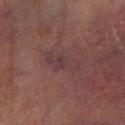Q: Is there a histopathology result?
A: no biopsy performed (imaged during a skin exam)
Q: Where on the body is the lesion?
A: the right lower leg
Q: How large is the lesion?
A: about 4.5 mm
Q: What is the imaging modality?
A: total-body-photography crop, ~15 mm field of view
Q: Patient demographics?
A: male, aged 73 to 77
Q: What lighting was used for the tile?
A: cross-polarized